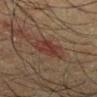A male patient, aged 58–62.
On the left lower leg.
The tile uses cross-polarized illumination.
This image is a 15 mm lesion crop taken from a total-body photograph.
Longest diameter approximately 4 mm.